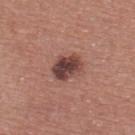<case>
  <biopsy_status>not biopsied; imaged during a skin examination</biopsy_status>
  <lighting>white-light</lighting>
  <image>
    <source>total-body photography crop</source>
    <field_of_view_mm>15</field_of_view_mm>
  </image>
  <site>upper back</site>
  <automated_metrics>
    <area_mm2_approx>8.5</area_mm2_approx>
    <eccentricity>0.75</eccentricity>
    <shape_asymmetry>0.2</shape_asymmetry>
    <cielab_L>42</cielab_L>
    <cielab_a>22</cielab_a>
    <cielab_b>22</cielab_b>
    <vs_skin_darker_L>15.0</vs_skin_darker_L>
    <vs_skin_contrast_norm>11.0</vs_skin_contrast_norm>
    <nevus_likeness_0_100>40</nevus_likeness_0_100>
    <lesion_detection_confidence_0_100>100</lesion_detection_confidence_0_100>
  </automated_metrics>
  <patient>
    <sex>male</sex>
    <age_approx>40</age_approx>
  </patient>
  <lesion_size>
    <long_diameter_mm_approx>4.0</long_diameter_mm_approx>
  </lesion_size>
</case>